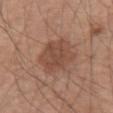follow-up = imaged on a skin check; not biopsied | location = the right upper arm | lesion size = ~5.5 mm (longest diameter) | image source = ~15 mm tile from a whole-body skin photo | patient = male, approximately 60 years of age | tile lighting = white-light | image-analysis metrics = a lesion color around L≈47 a*≈21 b*≈28 in CIELAB, roughly 9 lightness units darker than nearby skin, and a normalized lesion–skin contrast near 6.5; border irregularity of about 2.5 on a 0–10 scale, internal color variation of about 3 on a 0–10 scale, and radial color variation of about 1; an automated nevus-likeness rating near 5 out of 100.